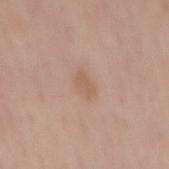{
  "biopsy_status": "not biopsied; imaged during a skin examination",
  "patient": {
    "sex": "female",
    "age_approx": 65
  },
  "lighting": "white-light",
  "automated_metrics": {
    "area_mm2_approx": 3.5,
    "eccentricity": 0.75,
    "shape_asymmetry": 0.25,
    "vs_skin_contrast_norm": 5.5
  },
  "image": {
    "source": "total-body photography crop",
    "field_of_view_mm": 15
  },
  "lesion_size": {
    "long_diameter_mm_approx": 2.5
  },
  "site": "back"
}